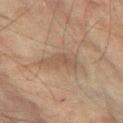• follow-up · no biopsy performed (imaged during a skin exam)
• tile lighting · cross-polarized illumination
• image-analysis metrics · a border-irregularity rating of about 4/10, a within-lesion color-variation index near 2/10, and radial color variation of about 0.5
• patient · male, in their mid- to late 70s
• acquisition · ~15 mm crop, total-body skin-cancer survey
• location · the left forearm
• lesion diameter · about 5 mm